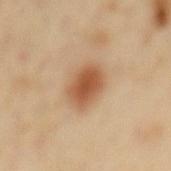Findings:
* follow-up — no biopsy performed (imaged during a skin exam)
* image — 15 mm crop, total-body photography
* image-analysis metrics — a footprint of about 11 mm², an outline eccentricity of about 0.75 (0 = round, 1 = elongated), and a shape-asymmetry score of about 0.15 (0 = symmetric); an automated nevus-likeness rating near 100 out of 100
* subject — male, aged approximately 65
* body site — the back